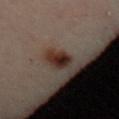Case summary:
– workup — catalogued during a skin exam; not biopsied
– lesion size — about 3.5 mm
– patient — female, roughly 40 years of age
– location — the upper back
– TBP lesion metrics — a nevus-likeness score of about 100/100
– acquisition — 15 mm crop, total-body photography